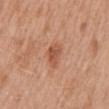Q: Was this lesion biopsied?
A: no biopsy performed (imaged during a skin exam)
Q: What did automated image analysis measure?
A: a mean CIELAB color near L≈54 a*≈26 b*≈33 and a lesion–skin lightness drop of about 10; a classifier nevus-likeness of about 70/100 and a lesion-detection confidence of about 100/100
Q: What kind of image is this?
A: ~15 mm tile from a whole-body skin photo
Q: How large is the lesion?
A: about 3 mm
Q: What lighting was used for the tile?
A: white-light illumination
Q: Lesion location?
A: the mid back
Q: Who is the patient?
A: female, aged 38 to 42